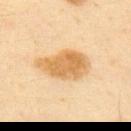This lesion was catalogued during total-body skin photography and was not selected for biopsy.
The tile uses cross-polarized illumination.
A region of skin cropped from a whole-body photographic capture, roughly 15 mm wide.
The lesion is located on the upper back.
An algorithmic analysis of the crop reported border irregularity of about 2.5 on a 0–10 scale, a within-lesion color-variation index near 3/10, and radial color variation of about 1. The software also gave a classifier nevus-likeness of about 90/100 and a lesion-detection confidence of about 100/100.
A male subject aged 33–37.